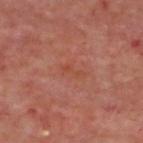Assessment: The lesion was photographed on a routine skin check and not biopsied; there is no pathology result. Background: Imaged with cross-polarized lighting. Measured at roughly 3 mm in maximum diameter. A patient aged approximately 55. The lesion is located on the upper back. A 15 mm crop from a total-body photograph taken for skin-cancer surveillance.